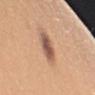Notes:
* biopsy status · catalogued during a skin exam; not biopsied
* subject · female, about 45 years old
* body site · the left upper arm
* acquisition · ~15 mm crop, total-body skin-cancer survey
* lesion size · ≈3.5 mm
* tile lighting · white-light
* image-analysis metrics · a footprint of about 8 mm², a shape eccentricity near 0.7, and a symmetry-axis asymmetry near 0.1; a mean CIELAB color near L≈56 a*≈20 b*≈29, a lesion–skin lightness drop of about 13, and a lesion-to-skin contrast of about 9.5 (normalized; higher = more distinct); a border-irregularity rating of about 1.5/10, a color-variation rating of about 5/10, and a peripheral color-asymmetry measure near 1.5